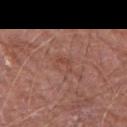workup = total-body-photography surveillance lesion; no biopsy
subject = male, aged around 70
site = the left upper arm
image source = ~15 mm crop, total-body skin-cancer survey